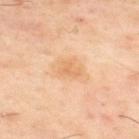The lesion was tiled from a total-body skin photograph and was not biopsied. This image is a 15 mm lesion crop taken from a total-body photograph. The total-body-photography lesion software estimated a lesion area of about 4 mm², an eccentricity of roughly 0.7, and a shape-asymmetry score of about 0.4 (0 = symmetric). Captured under cross-polarized illumination. A male patient roughly 60 years of age. The lesion is located on the upper back.